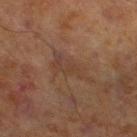{"image": {"source": "total-body photography crop", "field_of_view_mm": 15}, "lighting": "cross-polarized", "site": "left lower leg", "patient": {"sex": "male", "age_approx": 70}, "automated_metrics": {"border_irregularity_0_10": 6.5, "color_variation_0_10": 3.0}}